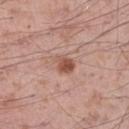Imaged during a routine full-body skin examination; the lesion was not biopsied and no histopathology is available. A male subject, about 55 years old. Imaged with white-light lighting. Automated image analysis of the tile measured an area of roughly 4.5 mm², an outline eccentricity of about 0.55 (0 = round, 1 = elongated), and two-axis asymmetry of about 0.3. And it measured a lesion color around L≈53 a*≈23 b*≈28 in CIELAB and about 11 CIELAB-L* units darker than the surrounding skin. And it measured a border-irregularity rating of about 2.5/10 and a color-variation rating of about 4/10. It also reported an automated nevus-likeness rating near 95 out of 100 and a lesion-detection confidence of about 100/100. Longest diameter approximately 2.5 mm. Cropped from a whole-body photographic skin survey; the tile spans about 15 mm. Located on the left thigh.